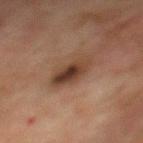<tbp_lesion>
<biopsy_status>not biopsied; imaged during a skin examination</biopsy_status>
<patient>
  <sex>male</sex>
  <age_approx>75</age_approx>
</patient>
<site>mid back</site>
<image>
  <source>total-body photography crop</source>
  <field_of_view_mm>15</field_of_view_mm>
</image>
<lesion_size>
  <long_diameter_mm_approx>5.0</long_diameter_mm_approx>
</lesion_size>
<lighting>cross-polarized</lighting>
</tbp_lesion>